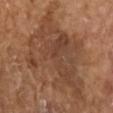Assessment:
Captured during whole-body skin photography for melanoma surveillance; the lesion was not biopsied.
Clinical summary:
The lesion is on the right forearm. A female patient, about 70 years old. A 15 mm close-up extracted from a 3D total-body photography capture. The tile uses cross-polarized illumination.